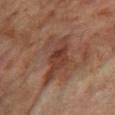Q: Is there a histopathology result?
A: imaged on a skin check; not biopsied
Q: What are the patient's age and sex?
A: female, aged approximately 55
Q: Where on the body is the lesion?
A: the right thigh
Q: Illumination type?
A: cross-polarized
Q: How was this image acquired?
A: total-body-photography crop, ~15 mm field of view